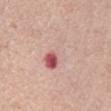Findings:
* image — 15 mm crop, total-body photography
* automated metrics — a lesion-detection confidence of about 100/100
* location — the abdomen
* patient — female, aged approximately 65
* lighting — white-light illumination